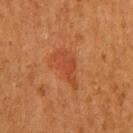Acquisition and patient details: A roughly 15 mm field-of-view crop from a total-body skin photograph. An algorithmic analysis of the crop reported an outline eccentricity of about 0.85 (0 = round, 1 = elongated) and two-axis asymmetry of about 0.4. The software also gave a mean CIELAB color near L≈38 a*≈25 b*≈33, about 6 CIELAB-L* units darker than the surrounding skin, and a normalized lesion–skin contrast near 5. And it measured a border-irregularity rating of about 4.5/10 and a within-lesion color-variation index near 3/10. From the right upper arm. The tile uses cross-polarized illumination. A female patient roughly 50 years of age. The lesion's longest dimension is about 5 mm.